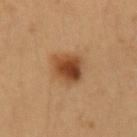workup: catalogued during a skin exam; not biopsied
patient: female, roughly 30 years of age
site: the left upper arm
image source: 15 mm crop, total-body photography